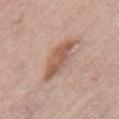patient = female, approximately 70 years of age
TBP lesion metrics = an average lesion color of about L≈57 a*≈19 b*≈29 (CIELAB) and a normalized lesion–skin contrast near 7.5; a border-irregularity rating of about 3/10, internal color variation of about 4 on a 0–10 scale, and a peripheral color-asymmetry measure near 1.5
lesion size = about 5.5 mm
lighting = white-light illumination
acquisition = ~15 mm tile from a whole-body skin photo
anatomic site = the left upper arm